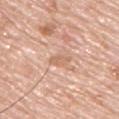workup = total-body-photography surveillance lesion; no biopsy.